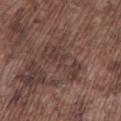Clinical impression:
Imaged during a routine full-body skin examination; the lesion was not biopsied and no histopathology is available.
Background:
The subject is a male aged around 75. Measured at roughly 5.5 mm in maximum diameter. A lesion tile, about 15 mm wide, cut from a 3D total-body photograph. The lesion is located on the left lower leg.